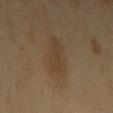patient — male, approximately 40 years of age
image source — ~15 mm tile from a whole-body skin photo
body site — the left upper arm
tile lighting — cross-polarized illumination
lesion diameter — ~4.5 mm (longest diameter)
automated metrics — an area of roughly 9 mm² and a shape eccentricity near 0.85